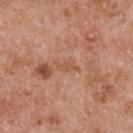follow-up: no biopsy performed (imaged during a skin exam)
automated metrics: a lesion area of about 2.5 mm², an outline eccentricity of about 0.9 (0 = round, 1 = elongated), and a shape-asymmetry score of about 0.4 (0 = symmetric); a lesion color around L≈54 a*≈23 b*≈33 in CIELAB and a normalized border contrast of about 4.5; peripheral color asymmetry of about 0
image: total-body-photography crop, ~15 mm field of view
anatomic site: the upper back
illumination: white-light
patient: male, roughly 55 years of age
size: about 3 mm